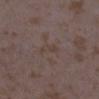Notes:
* biopsy status — no biopsy performed (imaged during a skin exam)
* image source — 15 mm crop, total-body photography
* subject — female, aged 33–37
* illumination — white-light illumination
* lesion diameter — ≈3 mm
* site — the leg